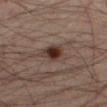{"biopsy_status": "not biopsied; imaged during a skin examination"}